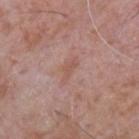This lesion was catalogued during total-body skin photography and was not selected for biopsy.
A male subject, aged 63 to 67.
Captured under white-light illumination.
A lesion tile, about 15 mm wide, cut from a 3D total-body photograph.
The lesion-visualizer software estimated roughly 6 lightness units darker than nearby skin and a normalized border contrast of about 5. And it measured border irregularity of about 5.5 on a 0–10 scale, a within-lesion color-variation index near 0.5/10, and radial color variation of about 0. The analysis additionally found a nevus-likeness score of about 0/100.
The recorded lesion diameter is about 3.5 mm.
From the upper back.